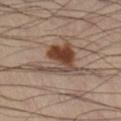Impression: Captured during whole-body skin photography for melanoma surveillance; the lesion was not biopsied. Acquisition and patient details: A 15 mm close-up tile from a total-body photography series done for melanoma screening. On the right lower leg. The lesion's longest dimension is about 6 mm. A male patient roughly 40 years of age.